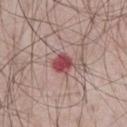workup: catalogued during a skin exam; not biopsied
tile lighting: white-light
subject: male, in their mid- to late 60s
image source: ~15 mm tile from a whole-body skin photo
lesion diameter: ~2.5 mm (longest diameter)
site: the chest